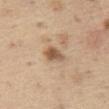workup = catalogued during a skin exam; not biopsied | acquisition = 15 mm crop, total-body photography | patient = male, in their 70s | lighting = white-light | site = the left thigh | diameter = ≈3 mm.